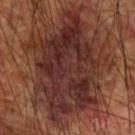Clinical impression:
This lesion was catalogued during total-body skin photography and was not selected for biopsy.
Background:
About 12 mm across. This is a cross-polarized tile. A close-up tile cropped from a whole-body skin photograph, about 15 mm across. The lesion is located on the arm. The subject is a male aged around 60.